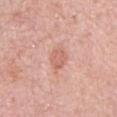The lesion was photographed on a routine skin check and not biopsied; there is no pathology result.
This image is a 15 mm lesion crop taken from a total-body photograph.
On the chest.
A male patient, roughly 75 years of age.